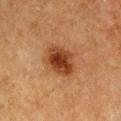follow-up = imaged on a skin check; not biopsied | anatomic site = the arm | lesion size = ≈4.5 mm | image source = 15 mm crop, total-body photography | tile lighting = cross-polarized | automated lesion analysis = a normalized border contrast of about 10; internal color variation of about 5 on a 0–10 scale and peripheral color asymmetry of about 1.5; a nevus-likeness score of about 100/100 and lesion-presence confidence of about 100/100 | patient = male, approximately 75 years of age.